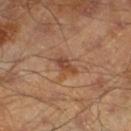* notes: total-body-photography surveillance lesion; no biopsy